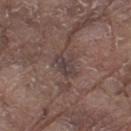Clinical impression: The lesion was photographed on a routine skin check and not biopsied; there is no pathology result. Image and clinical context: Imaged with white-light lighting. A region of skin cropped from a whole-body photographic capture, roughly 15 mm wide. A male subject in their 80s. On the left thigh. The recorded lesion diameter is about 3 mm. The lesion-visualizer software estimated an area of roughly 5.5 mm² and an outline eccentricity of about 0.7 (0 = round, 1 = elongated). The software also gave a border-irregularity rating of about 5.5/10, a within-lesion color-variation index near 2.5/10, and a peripheral color-asymmetry measure near 1.